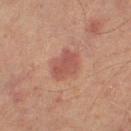Imaged during a routine full-body skin examination; the lesion was not biopsied and no histopathology is available. On the right thigh. Approximately 4 mm at its widest. A male patient, roughly 70 years of age. Cropped from a total-body skin-imaging series; the visible field is about 15 mm.